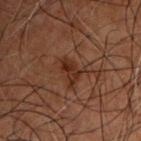{
  "biopsy_status": "not biopsied; imaged during a skin examination",
  "image": {
    "source": "total-body photography crop",
    "field_of_view_mm": 15
  },
  "patient": {
    "sex": "male",
    "age_approx": 50
  },
  "site": "chest",
  "lesion_size": {
    "long_diameter_mm_approx": 3.0
  },
  "automated_metrics": {
    "area_mm2_approx": 5.0,
    "eccentricity": 0.85,
    "shape_asymmetry": 0.25,
    "border_irregularity_0_10": 2.5,
    "color_variation_0_10": 3.5,
    "peripheral_color_asymmetry": 1.5
  },
  "lighting": "cross-polarized"
}